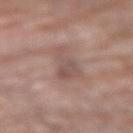Q: Is there a histopathology result?
A: no biopsy performed (imaged during a skin exam)
Q: How was this image acquired?
A: 15 mm crop, total-body photography
Q: Illumination type?
A: white-light illumination
Q: Automated lesion metrics?
A: a footprint of about 8.5 mm², a shape eccentricity near 0.95, and a symmetry-axis asymmetry near 0.5; a border-irregularity rating of about 6/10, a within-lesion color-variation index near 3/10, and peripheral color asymmetry of about 1
Q: What are the patient's age and sex?
A: female, aged 78–82
Q: What is the lesion's diameter?
A: ≈5.5 mm
Q: What is the anatomic site?
A: the left forearm